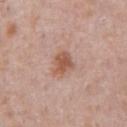Assessment:
Imaged during a routine full-body skin examination; the lesion was not biopsied and no histopathology is available.
Acquisition and patient details:
About 3 mm across. Captured under white-light illumination. The lesion is located on the chest. A 15 mm close-up extracted from a 3D total-body photography capture. A male subject aged 38–42.